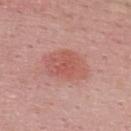The lesion was photographed on a routine skin check and not biopsied; there is no pathology result. From the upper back. A 15 mm crop from a total-body photograph taken for skin-cancer surveillance. A male patient aged 23–27. The total-body-photography lesion software estimated a lesion area of about 14 mm² and a shape-asymmetry score of about 0.15 (0 = symmetric). It also reported a mean CIELAB color near L≈56 a*≈28 b*≈27, about 8 CIELAB-L* units darker than the surrounding skin, and a normalized border contrast of about 6. The analysis additionally found border irregularity of about 2 on a 0–10 scale, internal color variation of about 3.5 on a 0–10 scale, and a peripheral color-asymmetry measure near 1.